The lesion is located on the front of the torso. Cropped from a total-body skin-imaging series; the visible field is about 15 mm. A female patient, approximately 70 years of age — 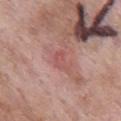Histopathological examination showed a lesion of indeterminate malignant potential: actinic keratosis.